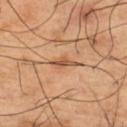Notes:
- notes · imaged on a skin check; not biopsied
- site · the right thigh
- size · ≈3 mm
- automated lesion analysis · border irregularity of about 3.5 on a 0–10 scale and peripheral color asymmetry of about 0.5; a classifier nevus-likeness of about 0/100 and a detector confidence of about 85 out of 100 that the crop contains a lesion
- image · 15 mm crop, total-body photography
- patient · male, aged 63–67